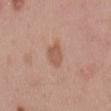The lesion was photographed on a routine skin check and not biopsied; there is no pathology result.
The lesion is on the mid back.
Automated image analysis of the tile measured a footprint of about 5.5 mm² and a symmetry-axis asymmetry near 0.3. And it measured an average lesion color of about L≈56 a*≈21 b*≈29 (CIELAB), about 8 CIELAB-L* units darker than the surrounding skin, and a normalized lesion–skin contrast near 6.5.
A male subject, aged around 50.
A 15 mm crop from a total-body photograph taken for skin-cancer surveillance.
Captured under white-light illumination.
Measured at roughly 3.5 mm in maximum diameter.